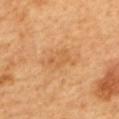Clinical impression: Imaged during a routine full-body skin examination; the lesion was not biopsied and no histopathology is available. Clinical summary: Measured at roughly 4.5 mm in maximum diameter. This is a cross-polarized tile. The subject is a female about 60 years old. A 15 mm close-up extracted from a 3D total-body photography capture. An algorithmic analysis of the crop reported a lesion area of about 7 mm², an outline eccentricity of about 0.85 (0 = round, 1 = elongated), and two-axis asymmetry of about 0.35. From the upper back.